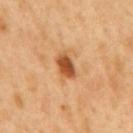biopsy status: no biopsy performed (imaged during a skin exam) | acquisition: ~15 mm crop, total-body skin-cancer survey | subject: male, aged 48–52 | TBP lesion metrics: a footprint of about 5.5 mm², a shape eccentricity near 0.8, and a symmetry-axis asymmetry near 0.25; a mean CIELAB color near L≈52 a*≈26 b*≈41 and a normalized lesion–skin contrast near 10.5 | body site: the mid back | diameter: ~3 mm (longest diameter).